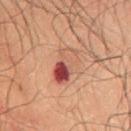<record>
<biopsy_status>not biopsied; imaged during a skin examination</biopsy_status>
<image>
  <source>total-body photography crop</source>
  <field_of_view_mm>15</field_of_view_mm>
</image>
<site>lower back</site>
<patient>
  <sex>male</sex>
  <age_approx>50</age_approx>
</patient>
<lesion_size>
  <long_diameter_mm_approx>5.0</long_diameter_mm_approx>
</lesion_size>
<automated_metrics>
  <area_mm2_approx>9.5</area_mm2_approx>
  <eccentricity>0.85</eccentricity>
  <shape_asymmetry>0.25</shape_asymmetry>
  <nevus_likeness_0_100>0</nevus_likeness_0_100>
  <lesion_detection_confidence_0_100>100</lesion_detection_confidence_0_100>
</automated_metrics>
</record>